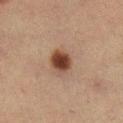Q: Was a biopsy performed?
A: no biopsy performed (imaged during a skin exam)
Q: How was this image acquired?
A: ~15 mm tile from a whole-body skin photo
Q: What are the patient's age and sex?
A: female, aged around 55
Q: How was the tile lit?
A: cross-polarized illumination
Q: How large is the lesion?
A: ~3 mm (longest diameter)
Q: What is the anatomic site?
A: the leg